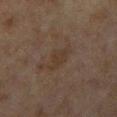Imaged during a routine full-body skin examination; the lesion was not biopsied and no histopathology is available.